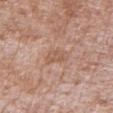The lesion was photographed on a routine skin check and not biopsied; there is no pathology result.
A 15 mm crop from a total-body photograph taken for skin-cancer surveillance.
The lesion is on the left lower leg.
A male subject aged around 60.